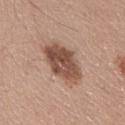notes = total-body-photography surveillance lesion; no biopsy | body site = the chest | lighting = white-light | subject = male, aged approximately 25 | image source = total-body-photography crop, ~15 mm field of view.